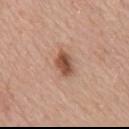No biopsy was performed on this lesion — it was imaged during a full skin examination and was not determined to be concerning. Located on the upper back. Automated image analysis of the tile measured a lesion area of about 6 mm², an outline eccentricity of about 0.7 (0 = round, 1 = elongated), and a shape-asymmetry score of about 0.2 (0 = symmetric). The subject is a male approximately 75 years of age. Imaged with white-light lighting. A region of skin cropped from a whole-body photographic capture, roughly 15 mm wide. About 3.5 mm across.